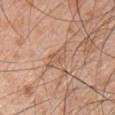biopsy status — catalogued during a skin exam; not biopsied | patient — male, aged around 50 | lesion diameter — ≈3.5 mm | lighting — white-light | automated lesion analysis — an eccentricity of roughly 0.9 and a shape-asymmetry score of about 0.4 (0 = symmetric); roughly 7 lightness units darker than nearby skin and a normalized lesion–skin contrast near 5 | acquisition — total-body-photography crop, ~15 mm field of view | anatomic site — the chest.